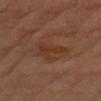Cropped from a whole-body photographic skin survey; the tile spans about 15 mm.
A female subject aged 68–72.
Located on the left upper arm.